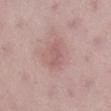Clinical summary:
On the right lower leg. This image is a 15 mm lesion crop taken from a total-body photograph. An algorithmic analysis of the crop reported a footprint of about 4 mm², an outline eccentricity of about 0.85 (0 = round, 1 = elongated), and two-axis asymmetry of about 0.35. It also reported a mean CIELAB color near L≈57 a*≈22 b*≈21, roughly 7 lightness units darker than nearby skin, and a normalized lesion–skin contrast near 4.5. It also reported a border-irregularity index near 3.5/10, a within-lesion color-variation index near 1/10, and peripheral color asymmetry of about 0.5. Longest diameter approximately 3 mm. A female patient, aged 38–42. Captured under white-light illumination.